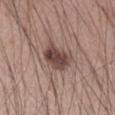biopsy_status: not biopsied; imaged during a skin examination